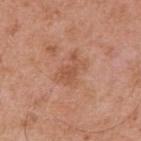{"site": "upper back", "automated_metrics": {"area_mm2_approx": 7.5, "shape_asymmetry": 0.4, "cielab_L": 54, "cielab_a": 24, "cielab_b": 33, "vs_skin_darker_L": 7.0, "nevus_likeness_0_100": 0}, "lesion_size": {"long_diameter_mm_approx": 4.0}, "lighting": "white-light", "image": {"source": "total-body photography crop", "field_of_view_mm": 15}, "patient": {"sex": "male", "age_approx": 55}}